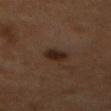Cropped from a whole-body photographic skin survey; the tile spans about 15 mm.
A male patient, in their 60s.
From the mid back.